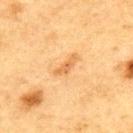  biopsy_status: not biopsied; imaged during a skin examination
  automated_metrics:
    border_irregularity_0_10: 4.0
    color_variation_0_10: 0.5
    peripheral_color_asymmetry: 0.0
    nevus_likeness_0_100: 10
    lesion_detection_confidence_0_100: 100
  patient:
    sex: male
    age_approx: 75
  site: upper back
  lighting: cross-polarized
  image:
    source: total-body photography crop
    field_of_view_mm: 15